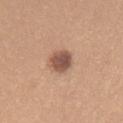The lesion was tiled from a total-body skin photograph and was not biopsied.